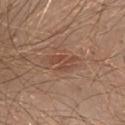This lesion was catalogued during total-body skin photography and was not selected for biopsy. About 3.5 mm across. Cropped from a total-body skin-imaging series; the visible field is about 15 mm. A male subject in their 40s. Automated image analysis of the tile measured a footprint of about 5 mm², an eccentricity of roughly 0.85, and two-axis asymmetry of about 0.4. The analysis additionally found an average lesion color of about L≈46 a*≈22 b*≈28 (CIELAB), a lesion–skin lightness drop of about 7, and a normalized border contrast of about 6. The software also gave a border-irregularity rating of about 5.5/10, internal color variation of about 1.5 on a 0–10 scale, and peripheral color asymmetry of about 0.5. From the abdomen.